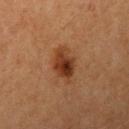  biopsy_status: not biopsied; imaged during a skin examination
  patient:
    sex: female
    age_approx: 40
  lesion_size:
    long_diameter_mm_approx: 4.5
  site: arm
  image:
    source: total-body photography crop
    field_of_view_mm: 15
  lighting: cross-polarized